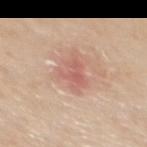Notes:
- tile lighting — white-light illumination
- image source — 15 mm crop, total-body photography
- lesion diameter — ~3 mm (longest diameter)
- patient — female, roughly 70 years of age
- body site — the upper back